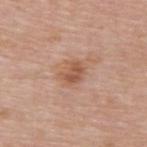  lighting: white-light
  patient:
    sex: male
    age_approx: 55
  site: upper back
  image:
    source: total-body photography crop
    field_of_view_mm: 15
  automated_metrics:
    cielab_L: 55
    cielab_a: 22
    cielab_b: 31
    vs_skin_darker_L: 10.0
    vs_skin_contrast_norm: 7.0
    border_irregularity_0_10: 2.5
    color_variation_0_10: 2.5